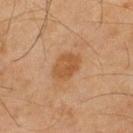• body site: the upper back
• tile lighting: cross-polarized
• automated metrics: a footprint of about 8 mm² and a shape eccentricity near 0.7; an average lesion color of about L≈42 a*≈18 b*≈32 (CIELAB), a lesion–skin lightness drop of about 7, and a lesion-to-skin contrast of about 7 (normalized; higher = more distinct)
• size: ≈3.5 mm
• image source: ~15 mm tile from a whole-body skin photo
• patient: male, roughly 45 years of age